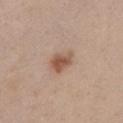{"biopsy_status": "not biopsied; imaged during a skin examination", "patient": {"sex": "female", "age_approx": 45}, "image": {"source": "total-body photography crop", "field_of_view_mm": 15}, "automated_metrics": {"eccentricity": 0.75, "shape_asymmetry": 0.25, "border_irregularity_0_10": 2.5, "color_variation_0_10": 3.5, "peripheral_color_asymmetry": 1.0, "nevus_likeness_0_100": 90, "lesion_detection_confidence_0_100": 100}, "site": "chest", "lighting": "white-light", "lesion_size": {"long_diameter_mm_approx": 3.5}}